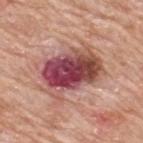No biopsy was performed on this lesion — it was imaged during a full skin examination and was not determined to be concerning.
This is a white-light tile.
The lesion is located on the upper back.
Cropped from a total-body skin-imaging series; the visible field is about 15 mm.
A male subject aged 78–82.
Measured at roughly 6.5 mm in maximum diameter.